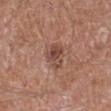| key | value |
|---|---|
| workup | imaged on a skin check; not biopsied |
| tile lighting | white-light |
| patient | male, aged 58 to 62 |
| acquisition | ~15 mm crop, total-body skin-cancer survey |
| anatomic site | the left lower leg |
| automated metrics | an area of roughly 5.5 mm², an outline eccentricity of about 0.65 (0 = round, 1 = elongated), and two-axis asymmetry of about 0.4; a border-irregularity index near 4/10, a color-variation rating of about 3.5/10, and radial color variation of about 1; a classifier nevus-likeness of about 45/100 and lesion-presence confidence of about 100/100 |
| lesion size | ≈3 mm |